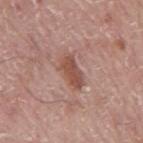Impression: Imaged during a routine full-body skin examination; the lesion was not biopsied and no histopathology is available. Clinical summary: A 15 mm crop from a total-body photograph taken for skin-cancer surveillance. Imaged with white-light lighting. A male subject roughly 75 years of age. The lesion is on the mid back.